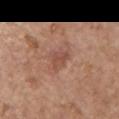Part of a total-body skin-imaging series; this lesion was reviewed on a skin check and was not flagged for biopsy. Cropped from a whole-body photographic skin survey; the tile spans about 15 mm. A female subject in their mid-60s. Located on the left upper arm.